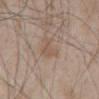workup=imaged on a skin check; not biopsied